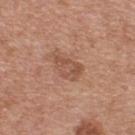The lesion was photographed on a routine skin check and not biopsied; there is no pathology result. A roughly 15 mm field-of-view crop from a total-body skin photograph. A female subject, about 40 years old. The lesion is on the upper back.